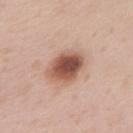The patient is a male in their mid-50s. Cropped from a total-body skin-imaging series; the visible field is about 15 mm. Located on the chest. The recorded lesion diameter is about 5 mm. Imaged with white-light lighting.